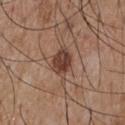Case summary:
– workup: catalogued during a skin exam; not biopsied